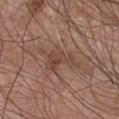Q: Was this lesion biopsied?
A: catalogued during a skin exam; not biopsied
Q: Where on the body is the lesion?
A: the chest
Q: Who is the patient?
A: male, about 65 years old
Q: Lesion size?
A: ~4 mm (longest diameter)
Q: How was this image acquired?
A: ~15 mm crop, total-body skin-cancer survey
Q: How was the tile lit?
A: white-light